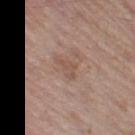<tbp_lesion>
<site>left thigh</site>
<automated_metrics>
  <area_mm2_approx>4.5</area_mm2_approx>
  <eccentricity>0.85</eccentricity>
  <shape_asymmetry>0.4</shape_asymmetry>
  <vs_skin_darker_L>6.0</vs_skin_darker_L>
  <vs_skin_contrast_norm>5.0</vs_skin_contrast_norm>
  <border_irregularity_0_10>4.5</border_irregularity_0_10>
  <color_variation_0_10>1.0</color_variation_0_10>
  <peripheral_color_asymmetry>0.5</peripheral_color_asymmetry>
</automated_metrics>
<image>
  <source>total-body photography crop</source>
  <field_of_view_mm>15</field_of_view_mm>
</image>
<patient>
  <sex>male</sex>
  <age_approx>60</age_approx>
</patient>
<lighting>white-light</lighting>
<lesion_size>
  <long_diameter_mm_approx>3.0</long_diameter_mm_approx>
</lesion_size>
</tbp_lesion>